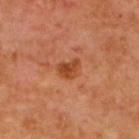Impression: The lesion was photographed on a routine skin check and not biopsied; there is no pathology result.